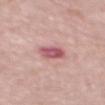Part of a total-body skin-imaging series; this lesion was reviewed on a skin check and was not flagged for biopsy.
A 15 mm crop from a total-body photograph taken for skin-cancer surveillance.
A male patient, aged around 70.
The recorded lesion diameter is about 3.5 mm.
On the back.